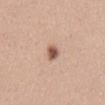workup: no biopsy performed (imaged during a skin exam)
patient: male, about 30 years old
diameter: about 2.5 mm
location: the mid back
illumination: white-light illumination
acquisition: ~15 mm crop, total-body skin-cancer survey